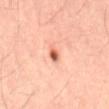Longest diameter approximately 2 mm. The subject is a male about 50 years old. The lesion is located on the mid back. Cropped from a whole-body photographic skin survey; the tile spans about 15 mm. Imaged with cross-polarized lighting. An algorithmic analysis of the crop reported a footprint of about 3 mm², an eccentricity of roughly 0.75, and two-axis asymmetry of about 0.3. The analysis additionally found a lesion color around L≈60 a*≈28 b*≈34 in CIELAB and a lesion–skin lightness drop of about 15. The analysis additionally found a within-lesion color-variation index near 6/10 and peripheral color asymmetry of about 2.